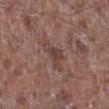| feature | finding |
|---|---|
| biopsy status | imaged on a skin check; not biopsied |
| lighting | white-light |
| acquisition | ~15 mm crop, total-body skin-cancer survey |
| patient | male, aged 53–57 |
| anatomic site | the right lower leg |